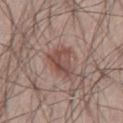A region of skin cropped from a whole-body photographic capture, roughly 15 mm wide.
The lesion is located on the left thigh.
Longest diameter approximately 4.5 mm.
The subject is a male aged around 55.
This is a white-light tile.
Automated tile analysis of the lesion measured a mean CIELAB color near L≈48 a*≈19 b*≈23 and roughly 9 lightness units darker than nearby skin. And it measured a classifier nevus-likeness of about 95/100 and a lesion-detection confidence of about 100/100.